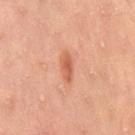biopsy status: catalogued during a skin exam; not biopsied | anatomic site: the right thigh | automated metrics: a footprint of about 3.5 mm², an eccentricity of roughly 0.95, and two-axis asymmetry of about 0.3; an average lesion color of about L≈59 a*≈29 b*≈35 (CIELAB) and about 11 CIELAB-L* units darker than the surrounding skin | image: 15 mm crop, total-body photography | patient: female, aged 33 to 37 | lesion diameter: about 3.5 mm | tile lighting: cross-polarized.